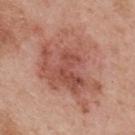| key | value |
|---|---|
| biopsy status | imaged on a skin check; not biopsied |
| tile lighting | white-light |
| patient | male, roughly 55 years of age |
| anatomic site | the upper back |
| size | ~9.5 mm (longest diameter) |
| image source | ~15 mm crop, total-body skin-cancer survey |